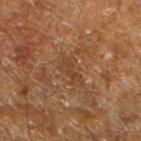Assessment:
Captured during whole-body skin photography for melanoma surveillance; the lesion was not biopsied.
Context:
The lesion is located on the leg. The tile uses cross-polarized illumination. A male patient, approximately 60 years of age. Longest diameter approximately 3 mm. A 15 mm crop from a total-body photograph taken for skin-cancer surveillance.